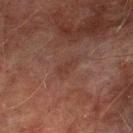Imaged during a routine full-body skin examination; the lesion was not biopsied and no histopathology is available.
A male patient, roughly 75 years of age.
This is a cross-polarized tile.
Cropped from a total-body skin-imaging series; the visible field is about 15 mm.
The lesion is located on the left lower leg.
The recorded lesion diameter is about 2.5 mm.